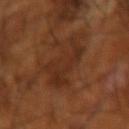notes: imaged on a skin check; not biopsied | lesion size: ≈5 mm | subject: male, aged around 65 | acquisition: ~15 mm crop, total-body skin-cancer survey | location: the right forearm | automated lesion analysis: an area of roughly 13 mm², a shape eccentricity near 0.7, and a symmetry-axis asymmetry near 0.55; a mean CIELAB color near L≈30 a*≈21 b*≈30, a lesion–skin lightness drop of about 6, and a normalized lesion–skin contrast near 6 | tile lighting: cross-polarized illumination.